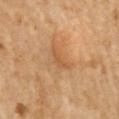patient:
  sex: female
  age_approx: 65
image:
  source: total-body photography crop
  field_of_view_mm: 15
lighting: cross-polarized
site: chest